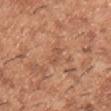Assessment:
Captured during whole-body skin photography for melanoma surveillance; the lesion was not biopsied.
Context:
Captured under white-light illumination. About 2.5 mm across. A 15 mm close-up extracted from a 3D total-body photography capture. An algorithmic analysis of the crop reported a shape eccentricity near 0.9 and two-axis asymmetry of about 0.35. The software also gave an average lesion color of about L≈53 a*≈23 b*≈33 (CIELAB) and roughly 7 lightness units darker than nearby skin. The software also gave radial color variation of about 0. A male subject, aged approximately 40. From the chest.